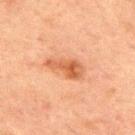The lesion was photographed on a routine skin check and not biopsied; there is no pathology result.
A male patient, in their 50s.
From the upper back.
A close-up tile cropped from a whole-body skin photograph, about 15 mm across.